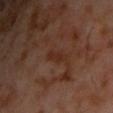Notes:
* notes: total-body-photography surveillance lesion; no biopsy